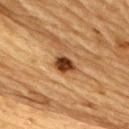The lesion was photographed on a routine skin check and not biopsied; there is no pathology result. Captured under cross-polarized illumination. A 15 mm close-up extracted from a 3D total-body photography capture. The lesion is on the upper back. A male subject aged 83 or over.